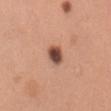No biopsy was performed on this lesion — it was imaged during a full skin examination and was not determined to be concerning. The subject is a female approximately 30 years of age. Captured under white-light illumination. Located on the lower back. A close-up tile cropped from a whole-body skin photograph, about 15 mm across. An algorithmic analysis of the crop reported an average lesion color of about L≈48 a*≈22 b*≈28 (CIELAB), a lesion–skin lightness drop of about 19, and a lesion-to-skin contrast of about 12.5 (normalized; higher = more distinct). The analysis additionally found a border-irregularity index near 1.5/10, a within-lesion color-variation index near 5.5/10, and radial color variation of about 1.5. The software also gave an automated nevus-likeness rating near 75 out of 100 and a lesion-detection confidence of about 100/100. The lesion's longest dimension is about 2.5 mm.